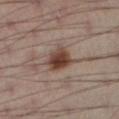Captured during whole-body skin photography for melanoma surveillance; the lesion was not biopsied. The lesion is on the left lower leg. The total-body-photography lesion software estimated an average lesion color of about L≈40 a*≈17 b*≈23 (CIELAB). Longest diameter approximately 3.5 mm. The tile uses cross-polarized illumination. A lesion tile, about 15 mm wide, cut from a 3D total-body photograph. The patient is a male aged 38–42.